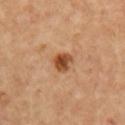The lesion was photographed on a routine skin check and not biopsied; there is no pathology result. The lesion's longest dimension is about 2.5 mm. A lesion tile, about 15 mm wide, cut from a 3D total-body photograph. Located on the right upper arm. A female subject, aged 43 to 47. Automated tile analysis of the lesion measured border irregularity of about 1.5 on a 0–10 scale, a color-variation rating of about 5/10, and radial color variation of about 1.5.